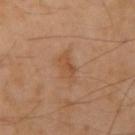Case summary:
* workup · no biopsy performed (imaged during a skin exam)
* patient · male, aged 48–52
* body site · the right upper arm
* image source · ~15 mm crop, total-body skin-cancer survey